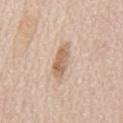The lesion was tiled from a total-body skin photograph and was not biopsied.
A male subject, aged around 65.
The lesion's longest dimension is about 4.5 mm.
This image is a 15 mm lesion crop taken from a total-body photograph.
Automated tile analysis of the lesion measured a nevus-likeness score of about 75/100.
The lesion is on the mid back.